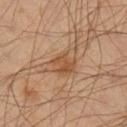workup — catalogued during a skin exam; not biopsied | lesion diameter — ~5 mm (longest diameter) | image-analysis metrics — an area of roughly 9.5 mm², an eccentricity of roughly 0.75, and a shape-asymmetry score of about 0.35 (0 = symmetric); a lesion color around L≈51 a*≈19 b*≈34 in CIELAB, about 8 CIELAB-L* units darker than the surrounding skin, and a normalized lesion–skin contrast near 6; a border-irregularity rating of about 4/10 and peripheral color asymmetry of about 1.5; an automated nevus-likeness rating near 15 out of 100 and a detector confidence of about 100 out of 100 that the crop contains a lesion | anatomic site — the leg | imaging modality — total-body-photography crop, ~15 mm field of view | lighting — cross-polarized | patient — male, in their mid- to late 40s.